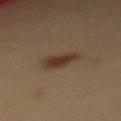Recorded during total-body skin imaging; not selected for excision or biopsy. From the mid back. A 15 mm close-up tile from a total-body photography series done for melanoma screening. The recorded lesion diameter is about 4.5 mm. The patient is a female in their mid- to late 30s. This is a cross-polarized tile.